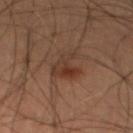Q: Was this lesion biopsied?
A: imaged on a skin check; not biopsied
Q: What kind of image is this?
A: 15 mm crop, total-body photography
Q: Automated lesion metrics?
A: roughly 7 lightness units darker than nearby skin; an automated nevus-likeness rating near 35 out of 100
Q: Patient demographics?
A: male, roughly 40 years of age
Q: Where on the body is the lesion?
A: the leg
Q: How large is the lesion?
A: ~4 mm (longest diameter)